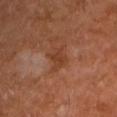Part of a total-body skin-imaging series; this lesion was reviewed on a skin check and was not flagged for biopsy. A lesion tile, about 15 mm wide, cut from a 3D total-body photograph. The recorded lesion diameter is about 3 mm. Imaged with cross-polarized lighting. The lesion-visualizer software estimated a footprint of about 5.5 mm², a shape eccentricity near 0.7, and a symmetry-axis asymmetry near 0.35. It also reported about 7 CIELAB-L* units darker than the surrounding skin and a normalized lesion–skin contrast near 6. And it measured a within-lesion color-variation index near 1.5/10. From the left upper arm. The subject is a male roughly 60 years of age.